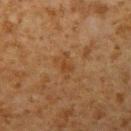The lesion was photographed on a routine skin check and not biopsied; there is no pathology result. The lesion is on the left upper arm. Approximately 3 mm at its widest. This is a cross-polarized tile. Automated tile analysis of the lesion measured a mean CIELAB color near L≈36 a*≈18 b*≈31. A 15 mm close-up extracted from a 3D total-body photography capture. The subject is a male in their 60s.